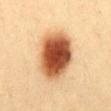Findings:
– follow-up — no biopsy performed (imaged during a skin exam)